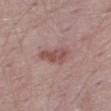{"biopsy_status": "not biopsied; imaged during a skin examination", "site": "right thigh", "image": {"source": "total-body photography crop", "field_of_view_mm": 15}, "patient": {"sex": "male", "age_approx": 50}}